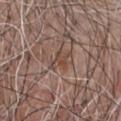size: ~3 mm (longest diameter)
subject: male, aged approximately 65
image-analysis metrics: a footprint of about 4 mm² and a shape-asymmetry score of about 0.4 (0 = symmetric); a border-irregularity rating of about 4/10, a color-variation rating of about 2/10, and radial color variation of about 0.5
anatomic site: the chest
acquisition: ~15 mm tile from a whole-body skin photo
tile lighting: white-light illumination